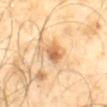biopsy status: imaged on a skin check; not biopsied
imaging modality: 15 mm crop, total-body photography
patient: male, approximately 65 years of age
tile lighting: cross-polarized illumination
size: about 3 mm
location: the back